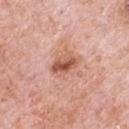<case>
<biopsy_status>not biopsied; imaged during a skin examination</biopsy_status>
<lighting>white-light</lighting>
<site>chest</site>
<patient>
  <sex>male</sex>
  <age_approx>80</age_approx>
</patient>
<image>
  <source>total-body photography crop</source>
  <field_of_view_mm>15</field_of_view_mm>
</image>
</case>